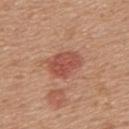Clinical impression:
Captured during whole-body skin photography for melanoma surveillance; the lesion was not biopsied.
Background:
The subject is a female approximately 55 years of age. This image is a 15 mm lesion crop taken from a total-body photograph. The lesion is located on the upper back. The tile uses white-light illumination. Approximately 4 mm at its widest. Automated image analysis of the tile measured a lesion area of about 10 mm², an outline eccentricity of about 0.7 (0 = round, 1 = elongated), and a symmetry-axis asymmetry near 0.2. It also reported a lesion color around L≈52 a*≈27 b*≈29 in CIELAB, about 10 CIELAB-L* units darker than the surrounding skin, and a lesion-to-skin contrast of about 7 (normalized; higher = more distinct). And it measured border irregularity of about 2 on a 0–10 scale, a color-variation rating of about 3/10, and radial color variation of about 1. And it measured a nevus-likeness score of about 95/100 and lesion-presence confidence of about 100/100.